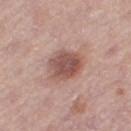follow-up: catalogued during a skin exam; not biopsied | patient: female, about 60 years old | lesion size: ≈5 mm | image: ~15 mm crop, total-body skin-cancer survey | site: the right thigh.